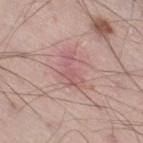Recorded during total-body skin imaging; not selected for excision or biopsy.
This is a white-light tile.
The lesion's longest dimension is about 3.5 mm.
On the right thigh.
A male subject, aged 53 to 57.
A lesion tile, about 15 mm wide, cut from a 3D total-body photograph.